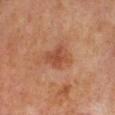notes = no biopsy performed (imaged during a skin exam) | lesion size = about 3 mm | location = the right lower leg | image = total-body-photography crop, ~15 mm field of view | subject = male, aged 68–72.